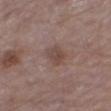Assessment:
The lesion was tiled from a total-body skin photograph and was not biopsied.
Background:
A 15 mm close-up extracted from a 3D total-body photography capture. The total-body-photography lesion software estimated an area of roughly 4.5 mm², an eccentricity of roughly 0.6, and a symmetry-axis asymmetry near 0.2. And it measured a normalized border contrast of about 6.5. And it measured a border-irregularity index near 2/10, a within-lesion color-variation index near 3.5/10, and peripheral color asymmetry of about 1.5. And it measured a nevus-likeness score of about 5/100 and a lesion-detection confidence of about 100/100. A male patient, aged approximately 80. The lesion is located on the right thigh. This is a white-light tile.